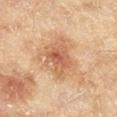Clinical impression:
Captured during whole-body skin photography for melanoma surveillance; the lesion was not biopsied.
Context:
A female subject, in their 60s. About 6 mm across. The lesion is located on the right lower leg. A 15 mm crop from a total-body photograph taken for skin-cancer surveillance. Automated tile analysis of the lesion measured a footprint of about 15 mm², an eccentricity of roughly 0.75, and two-axis asymmetry of about 0.25. The analysis additionally found an average lesion color of about L≈55 a*≈20 b*≈33 (CIELAB), roughly 10 lightness units darker than nearby skin, and a lesion-to-skin contrast of about 7 (normalized; higher = more distinct). And it measured a classifier nevus-likeness of about 40/100 and a lesion-detection confidence of about 100/100.